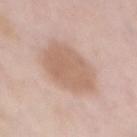Impression: Captured during whole-body skin photography for melanoma surveillance; the lesion was not biopsied. Acquisition and patient details: Located on the chest. A female subject, aged around 65. The tile uses white-light illumination. About 6 mm across. A close-up tile cropped from a whole-body skin photograph, about 15 mm across.